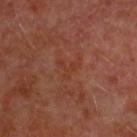workup — no biopsy performed (imaged during a skin exam) | image — total-body-photography crop, ~15 mm field of view | lighting — cross-polarized | site — the head or neck | TBP lesion metrics — a shape-asymmetry score of about 0.8 (0 = symmetric); a lesion color around L≈36 a*≈25 b*≈29 in CIELAB, about 4 CIELAB-L* units darker than the surrounding skin, and a normalized border contrast of about 5; border irregularity of about 8.5 on a 0–10 scale, a within-lesion color-variation index near 0/10, and peripheral color asymmetry of about 0; an automated nevus-likeness rating near 0 out of 100 and a detector confidence of about 100 out of 100 that the crop contains a lesion | subject — male, aged 58 to 62 | lesion diameter — ~2.5 mm (longest diameter).